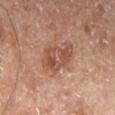{
  "biopsy_status": "not biopsied; imaged during a skin examination",
  "patient": {
    "sex": "male",
    "age_approx": 65
  },
  "image": {
    "source": "total-body photography crop",
    "field_of_view_mm": 15
  },
  "site": "leg",
  "automated_metrics": {
    "cielab_L": 48,
    "cielab_a": 21,
    "cielab_b": 29,
    "vs_skin_darker_L": 9.0,
    "vs_skin_contrast_norm": 7.0,
    "color_variation_0_10": 5.5,
    "nevus_likeness_0_100": 5
  },
  "lesion_size": {
    "long_diameter_mm_approx": 5.0
  }
}